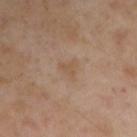Imaged during a routine full-body skin examination; the lesion was not biopsied and no histopathology is available.
Cropped from a whole-body photographic skin survey; the tile spans about 15 mm.
About 2.5 mm across.
A male subject, aged approximately 55.
Located on the right upper arm.
Captured under cross-polarized illumination.
An algorithmic analysis of the crop reported an area of roughly 3.5 mm² and an eccentricity of roughly 0.5. The software also gave an average lesion color of about L≈53 a*≈16 b*≈30 (CIELAB) and a normalized border contrast of about 5. The analysis additionally found a border-irregularity index near 4.5/10, a within-lesion color-variation index near 1.5/10, and peripheral color asymmetry of about 0.5. The analysis additionally found a classifier nevus-likeness of about 0/100.